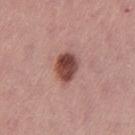Case summary:
• workup · no biopsy performed (imaged during a skin exam)
• size · about 4 mm
• imaging modality · ~15 mm crop, total-body skin-cancer survey
• body site · the left thigh
• patient · female, aged 53–57